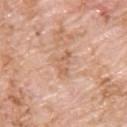On the upper back. A close-up tile cropped from a whole-body skin photograph, about 15 mm across. Longest diameter approximately 3 mm. A male patient aged 58 to 62. The tile uses white-light illumination. The lesion-visualizer software estimated a lesion area of about 4 mm² and an eccentricity of roughly 0.85. The analysis additionally found an average lesion color of about L≈62 a*≈21 b*≈33 (CIELAB), a lesion–skin lightness drop of about 8, and a normalized lesion–skin contrast near 5.5. And it measured a border-irregularity rating of about 7.5/10 and a peripheral color-asymmetry measure near 0. The analysis additionally found lesion-presence confidence of about 100/100.